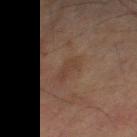Impression: Captured during whole-body skin photography for melanoma surveillance; the lesion was not biopsied. Context: A male patient, roughly 75 years of age. Located on the left thigh. Cropped from a whole-body photographic skin survey; the tile spans about 15 mm.